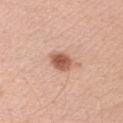<lesion>
  <biopsy_status>not biopsied; imaged during a skin examination</biopsy_status>
  <lesion_size>
    <long_diameter_mm_approx>3.0</long_diameter_mm_approx>
  </lesion_size>
  <site>right upper arm</site>
  <patient>
    <sex>female</sex>
    <age_approx>35</age_approx>
  </patient>
  <image>
    <source>total-body photography crop</source>
    <field_of_view_mm>15</field_of_view_mm>
  </image>
  <lighting>white-light</lighting>
</lesion>